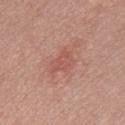{"biopsy_status": "not biopsied; imaged during a skin examination", "patient": {"sex": "male", "age_approx": 25}, "site": "chest", "image": {"source": "total-body photography crop", "field_of_view_mm": 15}}